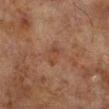A male patient, roughly 70 years of age. The lesion is on the right lower leg. A lesion tile, about 15 mm wide, cut from a 3D total-body photograph.